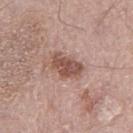Recorded during total-body skin imaging; not selected for excision or biopsy.
A 15 mm close-up tile from a total-body photography series done for melanoma screening.
On the left thigh.
A male subject, aged 73–77.